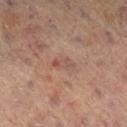<case>
<biopsy_status>not biopsied; imaged during a skin examination</biopsy_status>
<patient>
  <sex>female</sex>
  <age_approx>80</age_approx>
</patient>
<lesion_size>
  <long_diameter_mm_approx>3.0</long_diameter_mm_approx>
</lesion_size>
<site>left leg</site>
<lighting>cross-polarized</lighting>
<image>
  <source>total-body photography crop</source>
  <field_of_view_mm>15</field_of_view_mm>
</image>
</case>